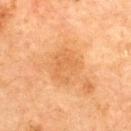Impression:
This lesion was catalogued during total-body skin photography and was not selected for biopsy.
Background:
A female subject, aged 58 to 62. Longest diameter approximately 4 mm. A close-up tile cropped from a whole-body skin photograph, about 15 mm across. An algorithmic analysis of the crop reported a shape-asymmetry score of about 0.25 (0 = symmetric). It also reported a classifier nevus-likeness of about 0/100 and a lesion-detection confidence of about 100/100. Imaged with cross-polarized lighting. Located on the back.